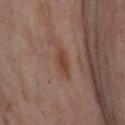<case>
  <biopsy_status>not biopsied; imaged during a skin examination</biopsy_status>
</case>